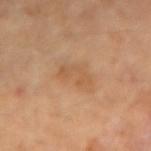Findings:
* notes · total-body-photography surveillance lesion; no biopsy
* diameter · ~4.5 mm (longest diameter)
* subject · female, about 60 years old
* body site · the left forearm
* automated metrics · a footprint of about 7.5 mm², an outline eccentricity of about 0.9 (0 = round, 1 = elongated), and two-axis asymmetry of about 0.35; border irregularity of about 4.5 on a 0–10 scale, internal color variation of about 2 on a 0–10 scale, and radial color variation of about 0.5
* acquisition · ~15 mm crop, total-body skin-cancer survey